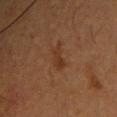Imaged during a routine full-body skin examination; the lesion was not biopsied and no histopathology is available. The lesion-visualizer software estimated a shape eccentricity near 0.9. It also reported internal color variation of about 2.5 on a 0–10 scale and peripheral color asymmetry of about 1. And it measured an automated nevus-likeness rating near 5 out of 100. Approximately 3 mm at its widest. The lesion is on the chest. A male subject in their mid- to late 50s. This image is a 15 mm lesion crop taken from a total-body photograph.